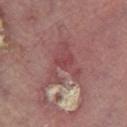Imaged during a routine full-body skin examination; the lesion was not biopsied and no histopathology is available. A male subject approximately 70 years of age. A region of skin cropped from a whole-body photographic capture, roughly 15 mm wide. Imaged with cross-polarized lighting. Located on the right lower leg.